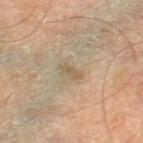<record>
  <biopsy_status>not biopsied; imaged during a skin examination</biopsy_status>
  <lighting>cross-polarized</lighting>
  <patient>
    <sex>male</sex>
    <age_approx>70</age_approx>
  </patient>
  <image>
    <source>total-body photography crop</source>
    <field_of_view_mm>15</field_of_view_mm>
  </image>
  <lesion_size>
    <long_diameter_mm_approx>2.5</long_diameter_mm_approx>
  </lesion_size>
  <site>right thigh</site>
</record>